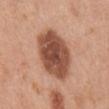Assessment: The lesion was photographed on a routine skin check and not biopsied; there is no pathology result. Image and clinical context: Captured under white-light illumination. Automated image analysis of the tile measured a lesion area of about 24 mm² and a shape eccentricity near 0.65. And it measured a border-irregularity index near 1.5/10, a color-variation rating of about 5/10, and peripheral color asymmetry of about 1.5. The lesion's longest dimension is about 6.5 mm. A roughly 15 mm field-of-view crop from a total-body skin photograph. Located on the mid back. A male patient, aged 28–32.